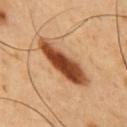Impression:
No biopsy was performed on this lesion — it was imaged during a full skin examination and was not determined to be concerning.
Background:
A male subject, aged 53 to 57. Captured under cross-polarized illumination. Measured at roughly 8 mm in maximum diameter. From the chest. A roughly 15 mm field-of-view crop from a total-body skin photograph.